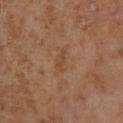Case summary:
– follow-up · total-body-photography surveillance lesion; no biopsy
– image source · 15 mm crop, total-body photography
– size · about 2.5 mm
– tile lighting · cross-polarized illumination
– automated lesion analysis · an area of roughly 2.5 mm², a shape eccentricity near 0.95, and a symmetry-axis asymmetry near 0.45; a normalized lesion–skin contrast near 5
– patient · male, roughly 70 years of age
– location · the right lower leg